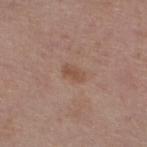Recorded during total-body skin imaging; not selected for excision or biopsy.
The tile uses white-light illumination.
Cropped from a total-body skin-imaging series; the visible field is about 15 mm.
A female subject, aged around 55.
Approximately 2.5 mm at its widest.
On the left lower leg.